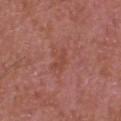Q: Was this lesion biopsied?
A: imaged on a skin check; not biopsied
Q: Lesion size?
A: ~2.5 mm (longest diameter)
Q: What are the patient's age and sex?
A: male, in their 40s
Q: What lighting was used for the tile?
A: white-light
Q: Where on the body is the lesion?
A: the head or neck
Q: What kind of image is this?
A: 15 mm crop, total-body photography